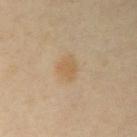| key | value |
|---|---|
| follow-up | total-body-photography surveillance lesion; no biopsy |
| tile lighting | cross-polarized illumination |
| lesion size | ~3 mm (longest diameter) |
| imaging modality | ~15 mm crop, total-body skin-cancer survey |
| body site | the right upper arm |
| subject | male, aged 48–52 |